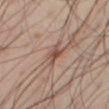Part of a total-body skin-imaging series; this lesion was reviewed on a skin check and was not flagged for biopsy.
A male subject, aged 48–52.
The tile uses cross-polarized illumination.
A close-up tile cropped from a whole-body skin photograph, about 15 mm across.
The recorded lesion diameter is about 3 mm.
From the right thigh.